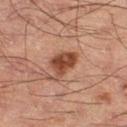| field | value |
|---|---|
| workup | imaged on a skin check; not biopsied |